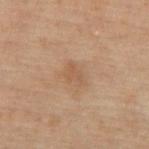Q: Is there a histopathology result?
A: no biopsy performed (imaged during a skin exam)